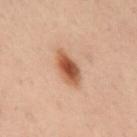This image is a 15 mm lesion crop taken from a total-body photograph. Automated image analysis of the tile measured a lesion color around L≈45 a*≈21 b*≈30 in CIELAB, a lesion–skin lightness drop of about 13, and a lesion-to-skin contrast of about 10.5 (normalized; higher = more distinct). The software also gave a border-irregularity index near 2/10, a color-variation rating of about 4.5/10, and radial color variation of about 1. The lesion's longest dimension is about 4 mm. On the mid back. A female patient, approximately 55 years of age.